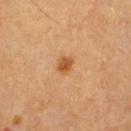The lesion was tiled from a total-body skin photograph and was not biopsied. A 15 mm close-up extracted from a 3D total-body photography capture. Located on the chest. The lesion-visualizer software estimated an area of roughly 4 mm² and an outline eccentricity of about 0.5 (0 = round, 1 = elongated). The analysis additionally found a border-irregularity rating of about 1.5/10, a color-variation rating of about 2.5/10, and a peripheral color-asymmetry measure near 1. It also reported a nevus-likeness score of about 95/100. A male patient, about 60 years old.